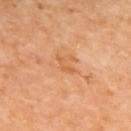<lesion>
  <site>upper back</site>
  <image>
    <source>total-body photography crop</source>
    <field_of_view_mm>15</field_of_view_mm>
  </image>
  <patient>
    <sex>female</sex>
    <age_approx>50</age_approx>
  </patient>
  <lighting>cross-polarized</lighting>
  <lesion_size>
    <long_diameter_mm_approx>2.5</long_diameter_mm_approx>
  </lesion_size>
</lesion>